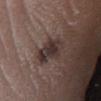Acquisition and patient details:
The lesion is on the right lower leg. This is a white-light tile. Cropped from a whole-body photographic skin survey; the tile spans about 15 mm. A male patient, in their mid- to late 30s. Longest diameter approximately 4 mm.